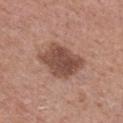Clinical impression: Imaged during a routine full-body skin examination; the lesion was not biopsied and no histopathology is available. Acquisition and patient details: Imaged with white-light lighting. A female patient aged around 50. Measured at roughly 5.5 mm in maximum diameter. From the chest. A 15 mm close-up tile from a total-body photography series done for melanoma screening. Automated image analysis of the tile measured a lesion–skin lightness drop of about 13 and a normalized border contrast of about 9.5.